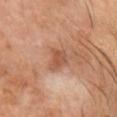This lesion was catalogued during total-body skin photography and was not selected for biopsy. A 15 mm close-up tile from a total-body photography series done for melanoma screening. The lesion's longest dimension is about 3 mm. This is a cross-polarized tile. The patient is a male aged 58 to 62. On the head or neck.